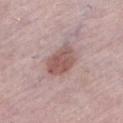Part of a total-body skin-imaging series; this lesion was reviewed on a skin check and was not flagged for biopsy.
This is a white-light tile.
The lesion is on the left lower leg.
Longest diameter approximately 4 mm.
A female subject in their mid- to late 60s.
The lesion-visualizer software estimated a mean CIELAB color near L≈54 a*≈20 b*≈21. The software also gave border irregularity of about 2 on a 0–10 scale.
This image is a 15 mm lesion crop taken from a total-body photograph.